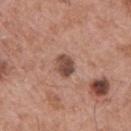  biopsy_status: not biopsied; imaged during a skin examination
  patient:
    sex: male
    age_approx: 65
  lighting: white-light
  site: upper back
  image:
    source: total-body photography crop
    field_of_view_mm: 15
  lesion_size:
    long_diameter_mm_approx: 2.5